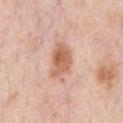biopsy status — imaged on a skin check; not biopsied
acquisition — total-body-photography crop, ~15 mm field of view
location — the chest
subject — male, aged around 50